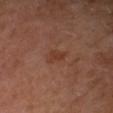Assessment: Imaged during a routine full-body skin examination; the lesion was not biopsied and no histopathology is available. Clinical summary: Imaged with cross-polarized lighting. This image is a 15 mm lesion crop taken from a total-body photograph. About 2.5 mm across. The lesion is located on the arm. The patient is a female in their mid- to late 50s.